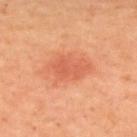No biopsy was performed on this lesion — it was imaged during a full skin examination and was not determined to be concerning. Longest diameter approximately 4 mm. A close-up tile cropped from a whole-body skin photograph, about 15 mm across. The tile uses cross-polarized illumination. Located on the upper back. Automated image analysis of the tile measured a lesion area of about 7 mm² and a symmetry-axis asymmetry near 0.35. The software also gave a lesion color around L≈60 a*≈33 b*≈38 in CIELAB. A female patient, aged 43 to 47.